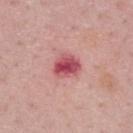workup = catalogued during a skin exam; not biopsied | subject = male, aged 48 to 52 | lighting = white-light illumination | acquisition = total-body-photography crop, ~15 mm field of view | site = the upper back | image-analysis metrics = a lesion area of about 7 mm²; about 15 CIELAB-L* units darker than the surrounding skin and a normalized border contrast of about 10; a border-irregularity index near 2/10, internal color variation of about 5.5 on a 0–10 scale, and a peripheral color-asymmetry measure near 2; a classifier nevus-likeness of about 0/100.